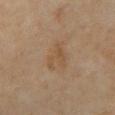Clinical impression:
Part of a total-body skin-imaging series; this lesion was reviewed on a skin check and was not flagged for biopsy.
Clinical summary:
Cropped from a total-body skin-imaging series; the visible field is about 15 mm. The lesion is located on the chest. This is a cross-polarized tile. The lesion-visualizer software estimated a lesion area of about 7 mm² and a shape-asymmetry score of about 0.4 (0 = symmetric). The analysis additionally found a lesion color around L≈48 a*≈15 b*≈31 in CIELAB, a lesion–skin lightness drop of about 5, and a lesion-to-skin contrast of about 5 (normalized; higher = more distinct). A male patient in their 60s.